The subject is a female in their mid- to late 80s or older · captured under white-light illumination · the lesion is on the chest · An algorithmic analysis of the crop reported an average lesion color of about L≈49 a*≈19 b*≈26 (CIELAB), about 15 CIELAB-L* units darker than the surrounding skin, and a normalized border contrast of about 10.5. It also reported border irregularity of about 6.5 on a 0–10 scale and a peripheral color-asymmetry measure near 4.5 · longest diameter approximately 13.5 mm · cropped from a total-body skin-imaging series; the visible field is about 15 mm:
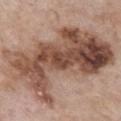On biopsy, histopathology showed a melanoma in situ.